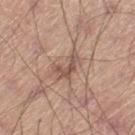Imaged during a routine full-body skin examination; the lesion was not biopsied and no histopathology is available. A 15 mm close-up extracted from a 3D total-body photography capture. The patient is a male aged 43–47. On the left thigh. Imaged with white-light lighting. Measured at roughly 3.5 mm in maximum diameter.